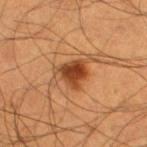Case summary:
– notes · total-body-photography surveillance lesion; no biopsy
– image source · 15 mm crop, total-body photography
– TBP lesion metrics · a lesion area of about 11 mm², a shape eccentricity near 0.8, and a shape-asymmetry score of about 0.4 (0 = symmetric); a border-irregularity rating of about 5.5/10, internal color variation of about 6.5 on a 0–10 scale, and radial color variation of about 2
– patient · male, approximately 55 years of age
– lighting · cross-polarized
– size · about 6 mm
– location · the right thigh